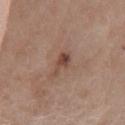biopsy_status: not biopsied; imaged during a skin examination
image:
  source: total-body photography crop
  field_of_view_mm: 15
site: chest
patient:
  sex: female
  age_approx: 40